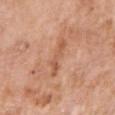{"biopsy_status": "not biopsied; imaged during a skin examination", "lesion_size": {"long_diameter_mm_approx": 4.5}, "patient": {"sex": "female", "age_approx": 70}, "image": {"source": "total-body photography crop", "field_of_view_mm": 15}, "site": "right upper arm"}